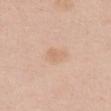biopsy status = total-body-photography surveillance lesion; no biopsy
lighting = white-light
size = ~3 mm (longest diameter)
anatomic site = the chest
image source = ~15 mm crop, total-body skin-cancer survey
patient = female, approximately 50 years of age